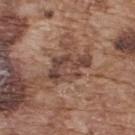No biopsy was performed on this lesion — it was imaged during a full skin examination and was not determined to be concerning. Imaged with white-light lighting. A male patient aged 73 to 77. This image is a 15 mm lesion crop taken from a total-body photograph. Automated image analysis of the tile measured a lesion–skin lightness drop of about 10 and a normalized lesion–skin contrast near 8.5. And it measured internal color variation of about 5 on a 0–10 scale. It also reported a nevus-likeness score of about 0/100. The lesion is on the upper back. About 5.5 mm across.